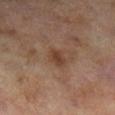{
  "biopsy_status": "not biopsied; imaged during a skin examination",
  "site": "right lower leg",
  "lighting": "cross-polarized",
  "patient": {
    "sex": "female",
    "age_approx": 60
  },
  "image": {
    "source": "total-body photography crop",
    "field_of_view_mm": 15
  },
  "lesion_size": {
    "long_diameter_mm_approx": 3.0
  }
}